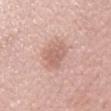{"biopsy_status": "not biopsied; imaged during a skin examination", "lesion_size": {"long_diameter_mm_approx": 3.0}, "patient": {"sex": "female", "age_approx": 50}, "image": {"source": "total-body photography crop", "field_of_view_mm": 15}, "site": "left forearm", "automated_metrics": {"border_irregularity_0_10": 2.0, "color_variation_0_10": 2.0, "peripheral_color_asymmetry": 0.5}}